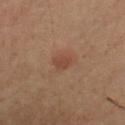{"biopsy_status": "not biopsied; imaged during a skin examination", "image": {"source": "total-body photography crop", "field_of_view_mm": 15}, "lesion_size": {"long_diameter_mm_approx": 2.5}, "automated_metrics": {"area_mm2_approx": 3.0, "eccentricity": 0.65, "shape_asymmetry": 0.3}, "lighting": "cross-polarized", "patient": {"sex": "male", "age_approx": 60}, "site": "arm"}